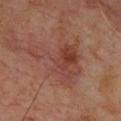Context: A male patient roughly 65 years of age. Approximately 7 mm at its widest. The tile uses cross-polarized illumination. The total-body-photography lesion software estimated a lesion color around L≈40 a*≈23 b*≈25 in CIELAB and a lesion–skin lightness drop of about 7. The lesion is located on the chest. A 15 mm close-up extracted from a 3D total-body photography capture.